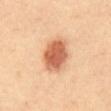follow-up — catalogued during a skin exam; not biopsied
lesion diameter — about 4.5 mm
acquisition — ~15 mm tile from a whole-body skin photo
lighting — cross-polarized
patient — female, aged around 35
location — the abdomen